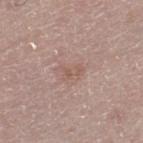image source = total-body-photography crop, ~15 mm field of view
body site = the left thigh
automated lesion analysis = an average lesion color of about L≈56 a*≈18 b*≈24 (CIELAB), about 6 CIELAB-L* units darker than the surrounding skin, and a normalized border contrast of about 5
lesion size = ~3 mm (longest diameter)
tile lighting = white-light
subject = male, roughly 65 years of age